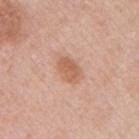The lesion was photographed on a routine skin check and not biopsied; there is no pathology result. A close-up tile cropped from a whole-body skin photograph, about 15 mm across. About 3.5 mm across. The lesion is located on the chest. The total-body-photography lesion software estimated an outline eccentricity of about 0.75 (0 = round, 1 = elongated) and two-axis asymmetry of about 0.15. It also reported a lesion color around L≈60 a*≈22 b*≈32 in CIELAB and roughly 9 lightness units darker than nearby skin. And it measured a detector confidence of about 100 out of 100 that the crop contains a lesion. A male subject aged 43–47.